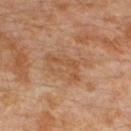Recorded during total-body skin imaging; not selected for excision or biopsy. A lesion tile, about 15 mm wide, cut from a 3D total-body photograph. A male subject approximately 30 years of age. The lesion is on the left lower leg. Automated tile analysis of the lesion measured a mean CIELAB color near L≈50 a*≈21 b*≈34 and a lesion-to-skin contrast of about 5.5 (normalized; higher = more distinct). And it measured an automated nevus-likeness rating near 0 out of 100 and lesion-presence confidence of about 100/100. Longest diameter approximately 5 mm.